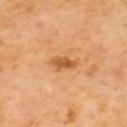This lesion was catalogued during total-body skin photography and was not selected for biopsy. About 3.5 mm across. This is a cross-polarized tile. The lesion is located on the upper back. The lesion-visualizer software estimated an area of roughly 4.5 mm², a shape eccentricity near 0.9, and a symmetry-axis asymmetry near 0.3. And it measured a lesion color around L≈55 a*≈26 b*≈43 in CIELAB and a lesion–skin lightness drop of about 11. It also reported a border-irregularity index near 3/10. A 15 mm close-up tile from a total-body photography series done for melanoma screening. A male subject aged around 70.